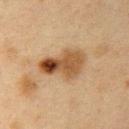The total-body-photography lesion software estimated a border-irregularity index near 4/10, a color-variation rating of about 10/10, and peripheral color asymmetry of about 4. The software also gave an automated nevus-likeness rating near 35 out of 100 and a lesion-detection confidence of about 100/100. Measured at roughly 5.5 mm in maximum diameter. The subject is a female aged around 40. Located on the right upper arm. Captured under cross-polarized illumination. A 15 mm crop from a total-body photograph taken for skin-cancer surveillance.